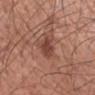Q: Is there a histopathology result?
A: total-body-photography surveillance lesion; no biopsy
Q: Who is the patient?
A: male, aged around 50
Q: What is the anatomic site?
A: the left forearm
Q: What lighting was used for the tile?
A: white-light illumination
Q: How was this image acquired?
A: total-body-photography crop, ~15 mm field of view
Q: How large is the lesion?
A: ~2.5 mm (longest diameter)